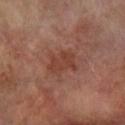Findings:
– notes — total-body-photography surveillance lesion; no biopsy
– illumination — cross-polarized
– location — the left arm
– patient — female, in their mid- to late 60s
– image-analysis metrics — an area of roughly 6.5 mm² and a shape-asymmetry score of about 0.3 (0 = symmetric); a classifier nevus-likeness of about 5/100 and a lesion-detection confidence of about 100/100
– acquisition — ~15 mm tile from a whole-body skin photo
– size — ≈3.5 mm